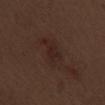Findings:
– workup · no biopsy performed (imaged during a skin exam)
– patient · male, about 70 years old
– diameter · ≈5 mm
– anatomic site · the right upper arm
– image source · total-body-photography crop, ~15 mm field of view
– illumination · white-light